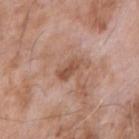Imaged during a routine full-body skin examination; the lesion was not biopsied and no histopathology is available.
The subject is a male aged approximately 75.
The tile uses white-light illumination.
The total-body-photography lesion software estimated a lesion area of about 4.5 mm², an outline eccentricity of about 0.85 (0 = round, 1 = elongated), and two-axis asymmetry of about 0.25. It also reported a border-irregularity index near 3/10, a within-lesion color-variation index near 2.5/10, and radial color variation of about 1. The software also gave a detector confidence of about 100 out of 100 that the crop contains a lesion.
On the right forearm.
A region of skin cropped from a whole-body photographic capture, roughly 15 mm wide.
Longest diameter approximately 3.5 mm.